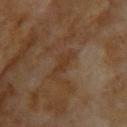follow-up: imaged on a skin check; not biopsied
subject: female, aged 58 to 62
body site: the head or neck
image source: total-body-photography crop, ~15 mm field of view